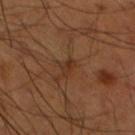Clinical impression:
Part of a total-body skin-imaging series; this lesion was reviewed on a skin check and was not flagged for biopsy.
Clinical summary:
A region of skin cropped from a whole-body photographic capture, roughly 15 mm wide. The subject is a male approximately 55 years of age. Automated image analysis of the tile measured a lesion–skin lightness drop of about 6. And it measured a nevus-likeness score of about 0/100 and a detector confidence of about 90 out of 100 that the crop contains a lesion. Captured under cross-polarized illumination. The lesion is located on the left lower leg.